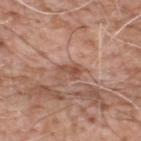The lesion was photographed on a routine skin check and not biopsied; there is no pathology result. The patient is a male approximately 60 years of age. The lesion is located on the upper back. A roughly 15 mm field-of-view crop from a total-body skin photograph. Imaged with white-light lighting. Approximately 3 mm at its widest. Automated image analysis of the tile measured an average lesion color of about L≈51 a*≈22 b*≈29 (CIELAB) and a lesion–skin lightness drop of about 9. It also reported a nevus-likeness score of about 0/100 and a detector confidence of about 100 out of 100 that the crop contains a lesion.